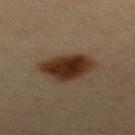<lesion>
  <biopsy_status>not biopsied; imaged during a skin examination</biopsy_status>
  <automated_metrics>
    <vs_skin_darker_L>13.0</vs_skin_darker_L>
    <border_irregularity_0_10>2.5</border_irregularity_0_10>
    <color_variation_0_10>4.0</color_variation_0_10>
    <peripheral_color_asymmetry>1.0</peripheral_color_asymmetry>
  </automated_metrics>
  <patient>
    <sex>female</sex>
    <age_approx>45</age_approx>
  </patient>
  <lighting>cross-polarized</lighting>
  <lesion_size>
    <long_diameter_mm_approx>5.5</long_diameter_mm_approx>
  </lesion_size>
  <image>
    <source>total-body photography crop</source>
    <field_of_view_mm>15</field_of_view_mm>
  </image>
  <site>upper back</site>
</lesion>